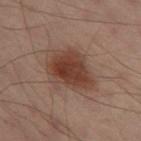No biopsy was performed on this lesion — it was imaged during a full skin examination and was not determined to be concerning. Imaged with cross-polarized lighting. The patient is a male in their 50s. From the left thigh. This image is a 15 mm lesion crop taken from a total-body photograph.